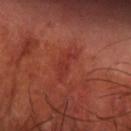Clinical summary: A male patient, roughly 70 years of age. This is a cross-polarized tile. The lesion-visualizer software estimated an outline eccentricity of about 0.9 (0 = round, 1 = elongated) and a symmetry-axis asymmetry near 0.35. The analysis additionally found a mean CIELAB color near L≈34 a*≈31 b*≈28, roughly 5 lightness units darker than nearby skin, and a normalized lesion–skin contrast near 4.5. It also reported a nevus-likeness score of about 0/100 and a lesion-detection confidence of about 100/100. From the right forearm. Longest diameter approximately 4 mm. Cropped from a total-body skin-imaging series; the visible field is about 15 mm.